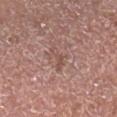biopsy_status: not biopsied; imaged during a skin examination
patient:
  sex: male
  age_approx: 55
image:
  source: total-body photography crop
  field_of_view_mm: 15
site: right lower leg
lighting: white-light